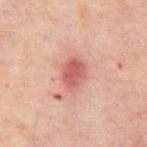<tbp_lesion>
  <biopsy_status>not biopsied; imaged during a skin examination</biopsy_status>
  <automated_metrics>
    <area_mm2_approx>8.0</area_mm2_approx>
    <shape_asymmetry>0.15</shape_asymmetry>
    <cielab_L>43</cielab_L>
    <cielab_a>23</cielab_a>
    <cielab_b>21</cielab_b>
    <vs_skin_darker_L>9.0</vs_skin_darker_L>
    <vs_skin_contrast_norm>7.5</vs_skin_contrast_norm>
    <border_irregularity_0_10>1.5</border_irregularity_0_10>
    <peripheral_color_asymmetry>1.0</peripheral_color_asymmetry>
    <nevus_likeness_0_100>55</nevus_likeness_0_100>
    <lesion_detection_confidence_0_100>100</lesion_detection_confidence_0_100>
  </automated_metrics>
  <lesion_size>
    <long_diameter_mm_approx>3.5</long_diameter_mm_approx>
  </lesion_size>
  <image>
    <source>total-body photography crop</source>
    <field_of_view_mm>15</field_of_view_mm>
  </image>
  <site>abdomen</site>
  <lighting>cross-polarized</lighting>
  <patient>
    <sex>male</sex>
    <age_approx>60</age_approx>
  </patient>
</tbp_lesion>